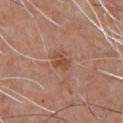* follow-up: catalogued during a skin exam; not biopsied
* patient: male, aged 53 to 57
* imaging modality: total-body-photography crop, ~15 mm field of view
* site: the front of the torso
* lesion diameter: about 2.5 mm